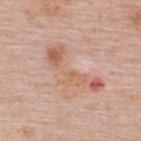<case>
  <biopsy_status>not biopsied; imaged during a skin examination</biopsy_status>
  <lesion_size>
    <long_diameter_mm_approx>7.5</long_diameter_mm_approx>
  </lesion_size>
  <lighting>white-light</lighting>
  <patient>
    <sex>female</sex>
    <age_approx>50</age_approx>
  </patient>
  <site>upper back</site>
  <automated_metrics>
    <area_mm2_approx>13.0</area_mm2_approx>
    <eccentricity>0.95</eccentricity>
    <shape_asymmetry>0.6</shape_asymmetry>
    <nevus_likeness_0_100>0</nevus_likeness_0_100>
    <lesion_detection_confidence_0_100>100</lesion_detection_confidence_0_100>
  </automated_metrics>
  <image>
    <source>total-body photography crop</source>
    <field_of_view_mm>15</field_of_view_mm>
  </image>
</case>